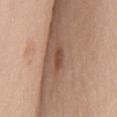Q: Is there a histopathology result?
A: no biopsy performed (imaged during a skin exam)
Q: Lesion location?
A: the mid back
Q: Lesion size?
A: about 2.5 mm
Q: What is the imaging modality?
A: ~15 mm crop, total-body skin-cancer survey
Q: Patient demographics?
A: female, roughly 50 years of age